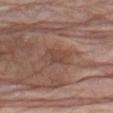  site: left upper arm
  patient:
    sex: male
    age_approx: 70
  lesion_size:
    long_diameter_mm_approx: 3.0
  lighting: white-light
  image:
    source: total-body photography crop
    field_of_view_mm: 15